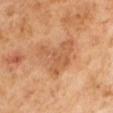Imaged during a routine full-body skin examination; the lesion was not biopsied and no histopathology is available. A male subject, aged around 65. A 15 mm close-up extracted from a 3D total-body photography capture.